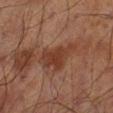| field | value |
|---|---|
| follow-up | no biopsy performed (imaged during a skin exam) |
| location | the left lower leg |
| illumination | cross-polarized illumination |
| acquisition | total-body-photography crop, ~15 mm field of view |
| subject | male, aged approximately 70 |